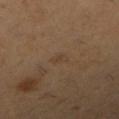No biopsy was performed on this lesion — it was imaged during a full skin examination and was not determined to be concerning.
A lesion tile, about 15 mm wide, cut from a 3D total-body photograph.
Longest diameter approximately 2 mm.
A female patient aged 53–57.
Located on the right lower leg.
Imaged with cross-polarized lighting.